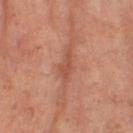workup: total-body-photography surveillance lesion; no biopsy | automated metrics: a lesion area of about 2.5 mm², an eccentricity of roughly 0.95, and a shape-asymmetry score of about 0.4 (0 = symmetric); a border-irregularity rating of about 5/10 and a within-lesion color-variation index near 0/10; a classifier nevus-likeness of about 0/100 and a lesion-detection confidence of about 55/100 | subject: female, in their 60s | image: 15 mm crop, total-body photography | anatomic site: the right thigh | illumination: cross-polarized.